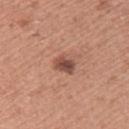biopsy_status: not biopsied; imaged during a skin examination
lighting: white-light
image:
  source: total-body photography crop
  field_of_view_mm: 15
automated_metrics:
  area_mm2_approx: 4.0
  eccentricity: 0.7
  shape_asymmetry: 0.25
  border_irregularity_0_10: 2.0
  peripheral_color_asymmetry: 1.5
site: left upper arm
patient:
  sex: female
  age_approx: 40
lesion_size:
  long_diameter_mm_approx: 2.5